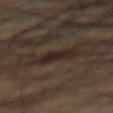Q: Was a biopsy performed?
A: imaged on a skin check; not biopsied
Q: How large is the lesion?
A: ≈4.5 mm
Q: How was this image acquired?
A: ~15 mm tile from a whole-body skin photo
Q: What are the patient's age and sex?
A: male, in their mid-60s
Q: What did automated image analysis measure?
A: a detector confidence of about 100 out of 100 that the crop contains a lesion
Q: Where on the body is the lesion?
A: the abdomen
Q: Illumination type?
A: cross-polarized